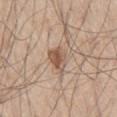Case summary:
* notes — catalogued during a skin exam; not biopsied
* patient — male, aged 58 to 62
* lighting — white-light illumination
* location — the right thigh
* diameter — about 3 mm
* image source — total-body-photography crop, ~15 mm field of view
* automated metrics — a footprint of about 6 mm², a shape eccentricity near 0.6, and two-axis asymmetry of about 0.35; a lesion color around L≈55 a*≈18 b*≈29 in CIELAB, about 11 CIELAB-L* units darker than the surrounding skin, and a lesion-to-skin contrast of about 8 (normalized; higher = more distinct); a border-irregularity rating of about 3/10 and a color-variation rating of about 5/10